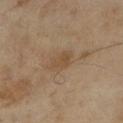Part of a total-body skin-imaging series; this lesion was reviewed on a skin check and was not flagged for biopsy.
A 15 mm close-up tile from a total-body photography series done for melanoma screening.
The lesion is located on the left lower leg.
Approximately 3.5 mm at its widest.
A female patient in their 60s.